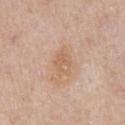workup: imaged on a skin check; not biopsied | lesion size: ≈3 mm | illumination: white-light illumination | location: the front of the torso | imaging modality: 15 mm crop, total-body photography | automated lesion analysis: a shape-asymmetry score of about 0.2 (0 = symmetric); a classifier nevus-likeness of about 0/100 and lesion-presence confidence of about 100/100 | patient: male, aged 58–62.